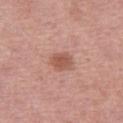The lesion was tiled from a total-body skin photograph and was not biopsied. Automated image analysis of the tile measured an area of roughly 5.5 mm², a shape eccentricity near 0.6, and two-axis asymmetry of about 0.2. The software also gave an average lesion color of about L≈55 a*≈24 b*≈28 (CIELAB) and roughly 10 lightness units darker than nearby skin. And it measured a classifier nevus-likeness of about 85/100 and a detector confidence of about 100 out of 100 that the crop contains a lesion. A female subject, aged approximately 70. From the right thigh. This is a white-light tile. Measured at roughly 3 mm in maximum diameter. Cropped from a total-body skin-imaging series; the visible field is about 15 mm.